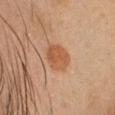Part of a total-body skin-imaging series; this lesion was reviewed on a skin check and was not flagged for biopsy. An algorithmic analysis of the crop reported an area of roughly 8 mm², an eccentricity of roughly 0.6, and two-axis asymmetry of about 0.15. The analysis additionally found an automated nevus-likeness rating near 85 out of 100 and a lesion-detection confidence of about 100/100. The recorded lesion diameter is about 3.5 mm. A 15 mm close-up extracted from a 3D total-body photography capture. A male patient aged 33–37. Captured under cross-polarized illumination. Located on the head or neck.